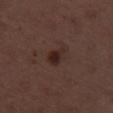workup: catalogued during a skin exam; not biopsied | illumination: white-light illumination | size: ≈3 mm | automated lesion analysis: two-axis asymmetry of about 0.3; a mean CIELAB color near L≈25 a*≈16 b*≈19, roughly 8 lightness units darker than nearby skin, and a normalized lesion–skin contrast near 8.5; border irregularity of about 2.5 on a 0–10 scale and radial color variation of about 1 | imaging modality: total-body-photography crop, ~15 mm field of view | location: the right thigh | subject: female, aged around 50.